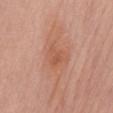notes — total-body-photography surveillance lesion; no biopsy
automated lesion analysis — internal color variation of about 2.5 on a 0–10 scale
image source — ~15 mm crop, total-body skin-cancer survey
lesion diameter — ≈3 mm
location — the chest
tile lighting — white-light
patient — female, in their mid- to late 60s